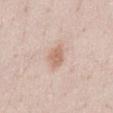Assessment:
The lesion was tiled from a total-body skin photograph and was not biopsied.
Clinical summary:
Imaged with white-light lighting. Located on the abdomen. The recorded lesion diameter is about 3.5 mm. Automated tile analysis of the lesion measured a lesion–skin lightness drop of about 9 and a normalized lesion–skin contrast near 6.5. It also reported a border-irregularity rating of about 2/10, a within-lesion color-variation index near 2/10, and a peripheral color-asymmetry measure near 0.5. The analysis additionally found an automated nevus-likeness rating near 90 out of 100 and a detector confidence of about 100 out of 100 that the crop contains a lesion. A male patient, aged 23 to 27. A roughly 15 mm field-of-view crop from a total-body skin photograph.